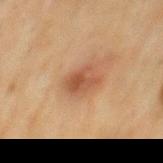Notes:
* biopsy status: catalogued during a skin exam; not biopsied
* acquisition: ~15 mm tile from a whole-body skin photo
* subject: male, aged 68–72
* location: the mid back
* lighting: cross-polarized
* automated metrics: an average lesion color of about L≈43 a*≈20 b*≈29 (CIELAB), about 9 CIELAB-L* units darker than the surrounding skin, and a normalized border contrast of about 7
* size: about 3 mm